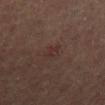notes = no biopsy performed (imaged during a skin exam); imaging modality = ~15 mm tile from a whole-body skin photo; lesion diameter = ≈2.5 mm; location = the back; tile lighting = cross-polarized illumination; patient = male, in their mid- to late 60s.